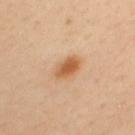Q: Was a biopsy performed?
A: imaged on a skin check; not biopsied